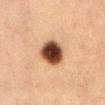{
  "biopsy_status": "not biopsied; imaged during a skin examination",
  "automated_metrics": {
    "lesion_detection_confidence_0_100": 100
  },
  "image": {
    "source": "total-body photography crop",
    "field_of_view_mm": 15
  },
  "patient": {
    "sex": "female",
    "age_approx": 40
  },
  "lesion_size": {
    "long_diameter_mm_approx": 4.0
  },
  "site": "abdomen"
}